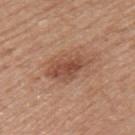Part of a total-body skin-imaging series; this lesion was reviewed on a skin check and was not flagged for biopsy.
This is a white-light tile.
The recorded lesion diameter is about 5.5 mm.
Automated tile analysis of the lesion measured a lesion area of about 12 mm², an eccentricity of roughly 0.8, and a shape-asymmetry score of about 0.25 (0 = symmetric). And it measured a mean CIELAB color near L≈50 a*≈22 b*≈30, about 10 CIELAB-L* units darker than the surrounding skin, and a normalized lesion–skin contrast near 7.5. It also reported a border-irregularity index near 3/10 and internal color variation of about 4.5 on a 0–10 scale.
A female patient roughly 50 years of age.
From the left upper arm.
A region of skin cropped from a whole-body photographic capture, roughly 15 mm wide.